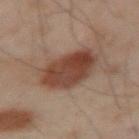Part of a total-body skin-imaging series; this lesion was reviewed on a skin check and was not flagged for biopsy. The total-body-photography lesion software estimated a lesion area of about 18 mm², a shape eccentricity near 0.7, and two-axis asymmetry of about 0.15. It also reported a lesion color around L≈33 a*≈17 b*≈23 in CIELAB, roughly 10 lightness units darker than nearby skin, and a lesion-to-skin contrast of about 9.5 (normalized; higher = more distinct). It also reported a border-irregularity index near 1.5/10, a within-lesion color-variation index near 4/10, and radial color variation of about 1.5. A male subject roughly 50 years of age. Approximately 6 mm at its widest. The lesion is located on the left upper arm. The tile uses cross-polarized illumination. A region of skin cropped from a whole-body photographic capture, roughly 15 mm wide.